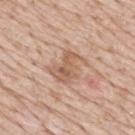Captured during whole-body skin photography for melanoma surveillance; the lesion was not biopsied.
From the mid back.
Cropped from a whole-body photographic skin survey; the tile spans about 15 mm.
The subject is a male roughly 80 years of age.